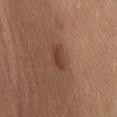An algorithmic analysis of the crop reported an area of roughly 3.5 mm² and a shape-asymmetry score of about 0.2 (0 = symmetric). The software also gave an average lesion color of about L≈40 a*≈22 b*≈29 (CIELAB), a lesion–skin lightness drop of about 9, and a normalized lesion–skin contrast near 7.5. It also reported an automated nevus-likeness rating near 80 out of 100 and a detector confidence of about 100 out of 100 that the crop contains a lesion. Longest diameter approximately 2.5 mm. A female subject in their mid-40s. This image is a 15 mm lesion crop taken from a total-body photograph. This is a white-light tile. The lesion is located on the abdomen.